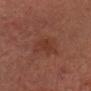Captured during whole-body skin photography for melanoma surveillance; the lesion was not biopsied.
Approximately 3 mm at its widest.
Located on the head or neck.
A male patient roughly 75 years of age.
A close-up tile cropped from a whole-body skin photograph, about 15 mm across.